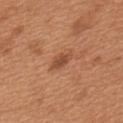notes: imaged on a skin check; not biopsied | lesion size: about 3 mm | image source: 15 mm crop, total-body photography | location: the upper back | patient: male, approximately 65 years of age.